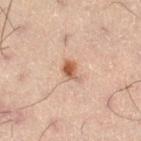biopsy_status: not biopsied; imaged during a skin examination
site: leg
image:
  source: total-body photography crop
  field_of_view_mm: 15
patient:
  sex: male
  age_approx: 50
lesion_size:
  long_diameter_mm_approx: 3.0